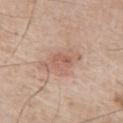Q: How was this image acquired?
A: ~15 mm tile from a whole-body skin photo
Q: Lesion size?
A: ≈5 mm
Q: Patient demographics?
A: male, aged approximately 65
Q: Where on the body is the lesion?
A: the right upper arm
Q: What lighting was used for the tile?
A: white-light